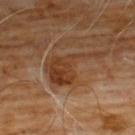Findings:
- notes · no biopsy performed (imaged during a skin exam)
- location · the chest
- acquisition · ~15 mm tile from a whole-body skin photo
- patient · male, approximately 60 years of age